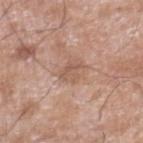Assessment: Captured during whole-body skin photography for melanoma surveillance; the lesion was not biopsied. Clinical summary: A male patient roughly 60 years of age. Measured at roughly 2.5 mm in maximum diameter. Located on the leg. The total-body-photography lesion software estimated a lesion area of about 4.5 mm², an outline eccentricity of about 0.5 (0 = round, 1 = elongated), and a symmetry-axis asymmetry near 0.4. And it measured a lesion color around L≈56 a*≈19 b*≈28 in CIELAB and roughly 8 lightness units darker than nearby skin. The analysis additionally found lesion-presence confidence of about 100/100. Imaged with white-light lighting. A region of skin cropped from a whole-body photographic capture, roughly 15 mm wide.